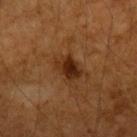| field | value |
|---|---|
| automated metrics | a lesion area of about 7 mm², a shape eccentricity near 0.65, and a shape-asymmetry score of about 0.2 (0 = symmetric); border irregularity of about 2.5 on a 0–10 scale, internal color variation of about 5.5 on a 0–10 scale, and radial color variation of about 2 |
| lesion diameter | ~3 mm (longest diameter) |
| illumination | cross-polarized |
| patient | male, aged 43 to 47 |
| acquisition | ~15 mm tile from a whole-body skin photo |
| location | the left forearm |